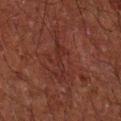Recorded during total-body skin imaging; not selected for excision or biopsy.
Cropped from a whole-body photographic skin survey; the tile spans about 15 mm.
Captured under cross-polarized illumination.
Located on the right forearm.
The patient is a male aged around 50.